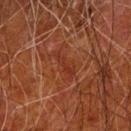This lesion was catalogued during total-body skin photography and was not selected for biopsy.
Automated tile analysis of the lesion measured a lesion area of about 3.5 mm², an eccentricity of roughly 0.95, and two-axis asymmetry of about 0.4. The software also gave an average lesion color of about L≈26 a*≈23 b*≈26 (CIELAB) and a normalized border contrast of about 5. The software also gave border irregularity of about 5.5 on a 0–10 scale, internal color variation of about 0 on a 0–10 scale, and a peripheral color-asymmetry measure near 0. It also reported a classifier nevus-likeness of about 0/100 and lesion-presence confidence of about 95/100.
The patient is a male aged 78 to 82.
Measured at roughly 3.5 mm in maximum diameter.
Captured under cross-polarized illumination.
A region of skin cropped from a whole-body photographic capture, roughly 15 mm wide.
The lesion is located on the left forearm.